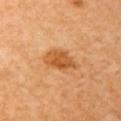<lesion>
<biopsy_status>not biopsied; imaged during a skin examination</biopsy_status>
<site>left upper arm</site>
<automated_metrics>
  <border_irregularity_0_10>3.0</border_irregularity_0_10>
  <color_variation_0_10>3.5</color_variation_0_10>
  <peripheral_color_asymmetry>1.5</peripheral_color_asymmetry>
</automated_metrics>
<lighting>cross-polarized</lighting>
<patient>
  <sex>female</sex>
  <age_approx>65</age_approx>
</patient>
<image>
  <source>total-body photography crop</source>
  <field_of_view_mm>15</field_of_view_mm>
</image>
</lesion>